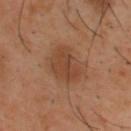Recorded during total-body skin imaging; not selected for excision or biopsy. Automated image analysis of the tile measured an average lesion color of about L≈42 a*≈21 b*≈31 (CIELAB) and a lesion-to-skin contrast of about 6.5 (normalized; higher = more distinct). The analysis additionally found a border-irregularity index near 3/10, a within-lesion color-variation index near 2.5/10, and radial color variation of about 1. The analysis additionally found a classifier nevus-likeness of about 75/100 and a detector confidence of about 100 out of 100 that the crop contains a lesion. A male patient, roughly 40 years of age. Approximately 4 mm at its widest. On the upper back. Captured under cross-polarized illumination. This image is a 15 mm lesion crop taken from a total-body photograph.